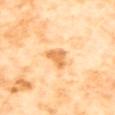Imaged during a routine full-body skin examination; the lesion was not biopsied and no histopathology is available.
Measured at roughly 2.5 mm in maximum diameter.
The total-body-photography lesion software estimated a footprint of about 5 mm² and an outline eccentricity of about 0.45 (0 = round, 1 = elongated). It also reported an automated nevus-likeness rating near 5 out of 100 and a lesion-detection confidence of about 100/100.
A 15 mm crop from a total-body photograph taken for skin-cancer surveillance.
From the mid back.
A female subject, in their mid-50s.
This is a cross-polarized tile.